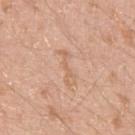| key | value |
|---|---|
| follow-up | total-body-photography surveillance lesion; no biopsy |
| illumination | white-light |
| acquisition | ~15 mm tile from a whole-body skin photo |
| site | the left upper arm |
| patient | male, roughly 20 years of age |
| diameter | ≈4 mm |